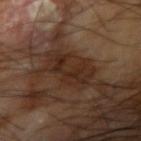<case>
<biopsy_status>not biopsied; imaged during a skin examination</biopsy_status>
<automated_metrics>
  <cielab_L>26</cielab_L>
  <cielab_a>17</cielab_a>
  <cielab_b>25</cielab_b>
  <vs_skin_darker_L>8.0</vs_skin_darker_L>
  <vs_skin_contrast_norm>9.0</vs_skin_contrast_norm>
</automated_metrics>
<site>right upper arm</site>
<image>
  <source>total-body photography crop</source>
  <field_of_view_mm>15</field_of_view_mm>
</image>
<patient>
  <sex>male</sex>
  <age_approx>65</age_approx>
</patient>
<lighting>cross-polarized</lighting>
<lesion_size>
  <long_diameter_mm_approx>5.0</long_diameter_mm_approx>
</lesion_size>
</case>